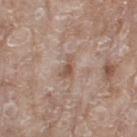Clinical impression:
The lesion was tiled from a total-body skin photograph and was not biopsied.
Background:
This image is a 15 mm lesion crop taken from a total-body photograph. This is a white-light tile. The total-body-photography lesion software estimated a lesion area of about 3.5 mm², an eccentricity of roughly 0.9, and a symmetry-axis asymmetry near 0.5. It also reported a mean CIELAB color near L≈53 a*≈17 b*≈26, roughly 10 lightness units darker than nearby skin, and a normalized lesion–skin contrast near 7. And it measured a border-irregularity rating of about 5/10, a within-lesion color-variation index near 1.5/10, and peripheral color asymmetry of about 0.5. A female subject in their mid- to late 70s. On the right thigh. Measured at roughly 3 mm in maximum diameter.